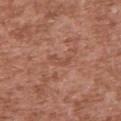Imaged during a routine full-body skin examination; the lesion was not biopsied and no histopathology is available. Measured at roughly 3 mm in maximum diameter. The lesion is located on the chest. A male patient, aged 43 to 47. A close-up tile cropped from a whole-body skin photograph, about 15 mm across. The tile uses white-light illumination.